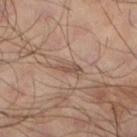Assessment: No biopsy was performed on this lesion — it was imaged during a full skin examination and was not determined to be concerning. Clinical summary: A male patient, approximately 65 years of age. A region of skin cropped from a whole-body photographic capture, roughly 15 mm wide. On the left lower leg.